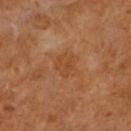Assessment: The lesion was photographed on a routine skin check and not biopsied; there is no pathology result. Image and clinical context: The lesion is on the right upper arm. A female patient, aged around 55. The lesion's longest dimension is about 3 mm. A region of skin cropped from a whole-body photographic capture, roughly 15 mm wide. Captured under cross-polarized illumination.